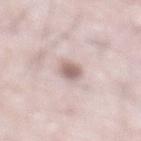<tbp_lesion>
<biopsy_status>not biopsied; imaged during a skin examination</biopsy_status>
<patient>
  <sex>male</sex>
  <age_approx>75</age_approx>
</patient>
<image>
  <source>total-body photography crop</source>
  <field_of_view_mm>15</field_of_view_mm>
</image>
<site>abdomen</site>
</tbp_lesion>